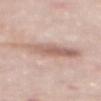{"biopsy_status": "not biopsied; imaged during a skin examination", "site": "mid back", "patient": {"sex": "female", "age_approx": 65}, "image": {"source": "total-body photography crop", "field_of_view_mm": 15}, "lesion_size": {"long_diameter_mm_approx": 7.0}, "lighting": "white-light", "automated_metrics": {"area_mm2_approx": 17.0, "eccentricity": 0.9, "border_irregularity_0_10": 3.5, "peripheral_color_asymmetry": 2.0, "nevus_likeness_0_100": 0, "lesion_detection_confidence_0_100": 100}}